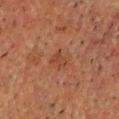notes: catalogued during a skin exam; not biopsied
tile lighting: cross-polarized illumination
site: the head or neck
patient: male, aged 58 to 62
imaging modality: ~15 mm tile from a whole-body skin photo